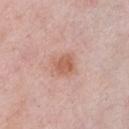notes = no biopsy performed (imaged during a skin exam); image source = 15 mm crop, total-body photography; location = the chest; subject = female, in their 60s; lighting = white-light illumination; lesion diameter = about 2.5 mm.